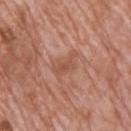{
  "biopsy_status": "not biopsied; imaged during a skin examination",
  "image": {
    "source": "total-body photography crop",
    "field_of_view_mm": 15
  },
  "lighting": "white-light",
  "lesion_size": {
    "long_diameter_mm_approx": 4.0
  },
  "automated_metrics": {
    "area_mm2_approx": 6.0,
    "eccentricity": 0.85,
    "vs_skin_darker_L": 7.0,
    "vs_skin_contrast_norm": 5.5,
    "lesion_detection_confidence_0_100": 100
  },
  "patient": {
    "sex": "male",
    "age_approx": 70
  },
  "site": "upper back"
}